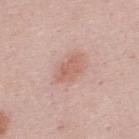Imaged during a routine full-body skin examination; the lesion was not biopsied and no histopathology is available.
About 4 mm across.
This image is a 15 mm lesion crop taken from a total-body photograph.
The lesion is located on the upper back.
Captured under white-light illumination.
An algorithmic analysis of the crop reported a border-irregularity index near 2.5/10, a within-lesion color-variation index near 2/10, and peripheral color asymmetry of about 0.5. The software also gave a detector confidence of about 100 out of 100 that the crop contains a lesion.
The subject is a male aged 23 to 27.